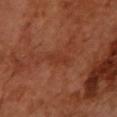Assessment: This lesion was catalogued during total-body skin photography and was not selected for biopsy. Background: About 3 mm across. Automated image analysis of the tile measured a border-irregularity index near 3/10, a within-lesion color-variation index near 0.5/10, and a peripheral color-asymmetry measure near 0. A region of skin cropped from a whole-body photographic capture, roughly 15 mm wide. A female subject aged 68–72. The lesion is located on the left forearm.